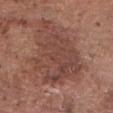  biopsy_status: not biopsied; imaged during a skin examination
  lighting: white-light
  automated_metrics:
    area_mm2_approx: 34.0
    eccentricity: 0.65
    shape_asymmetry: 0.4
    border_irregularity_0_10: 7.0
  image:
    source: total-body photography crop
    field_of_view_mm: 15
  patient:
    sex: male
    age_approx: 75
  site: head or neck
  lesion_size:
    long_diameter_mm_approx: 8.5